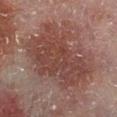Q: Was this lesion biopsied?
A: total-body-photography surveillance lesion; no biopsy
Q: How was this image acquired?
A: total-body-photography crop, ~15 mm field of view
Q: Who is the patient?
A: male, in their 60s
Q: How was the tile lit?
A: cross-polarized
Q: Automated lesion metrics?
A: a footprint of about 43 mm², an eccentricity of roughly 0.75, and a symmetry-axis asymmetry near 0.25; roughly 8 lightness units darker than nearby skin and a lesion-to-skin contrast of about 7 (normalized; higher = more distinct); a border-irregularity rating of about 4.5/10, a color-variation rating of about 4/10, and a peripheral color-asymmetry measure near 1.5
Q: What is the anatomic site?
A: the right lower leg
Q: What is the lesion's diameter?
A: ~10 mm (longest diameter)